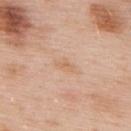Captured during whole-body skin photography for melanoma surveillance; the lesion was not biopsied.
Approximately 2.5 mm at its widest.
On the upper back.
A male subject, aged around 55.
Imaged with white-light lighting.
A 15 mm close-up tile from a total-body photography series done for melanoma screening.